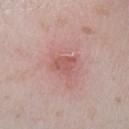follow-up: imaged on a skin check; not biopsied | subject: female, aged around 25 | automated lesion analysis: a lesion area of about 4 mm², an eccentricity of roughly 0.6, and a shape-asymmetry score of about 0.35 (0 = symmetric); a classifier nevus-likeness of about 0/100 and a lesion-detection confidence of about 100/100 | lighting: white-light illumination | image source: 15 mm crop, total-body photography | site: the left lower leg.